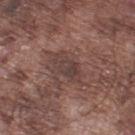Part of a total-body skin-imaging series; this lesion was reviewed on a skin check and was not flagged for biopsy.
Measured at roughly 4 mm in maximum diameter.
A male subject, about 75 years old.
This is a white-light tile.
A roughly 15 mm field-of-view crop from a total-body skin photograph.
From the leg.
The total-body-photography lesion software estimated a footprint of about 7 mm² and an eccentricity of roughly 0.85. The software also gave roughly 7 lightness units darker than nearby skin and a lesion-to-skin contrast of about 6.5 (normalized; higher = more distinct). The analysis additionally found border irregularity of about 3 on a 0–10 scale and peripheral color asymmetry of about 1.